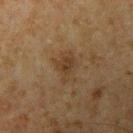From the left upper arm.
A region of skin cropped from a whole-body photographic capture, roughly 15 mm wide.
The patient is a male aged 58 to 62.